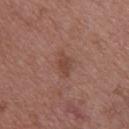workup=imaged on a skin check; not biopsied
image source=15 mm crop, total-body photography
illumination=white-light illumination
subject=female, approximately 60 years of age
size=≈3 mm
anatomic site=the upper back